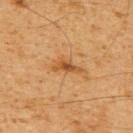notes: no biopsy performed (imaged during a skin exam)
patient: male, aged 58 to 62
location: the upper back
imaging modality: total-body-photography crop, ~15 mm field of view
size: ≈3.5 mm
tile lighting: cross-polarized